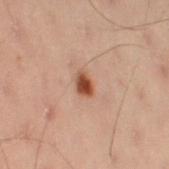Q: Is there a histopathology result?
A: total-body-photography surveillance lesion; no biopsy
Q: Patient demographics?
A: male, approximately 50 years of age
Q: Where on the body is the lesion?
A: the leg
Q: How was this image acquired?
A: 15 mm crop, total-body photography